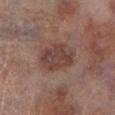follow-up: no biopsy performed (imaged during a skin exam); imaging modality: ~15 mm crop, total-body skin-cancer survey; illumination: cross-polarized illumination; size: ≈5 mm; anatomic site: the leg; patient: male, aged approximately 65.